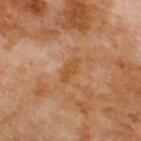Notes:
• follow-up — total-body-photography surveillance lesion; no biopsy
• subject — male, aged 68 to 72
• automated metrics — a lesion area of about 4.5 mm² and two-axis asymmetry of about 0.2; a lesion color around L≈51 a*≈23 b*≈39 in CIELAB and a normalized border contrast of about 6.5; a border-irregularity rating of about 2.5/10, a within-lesion color-variation index near 2/10, and a peripheral color-asymmetry measure near 1
• site — the back
• diameter — ≈3.5 mm
• imaging modality — ~15 mm crop, total-body skin-cancer survey